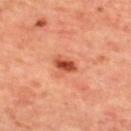• notes — total-body-photography surveillance lesion; no biopsy
• subject — female, in their mid-40s
• image — 15 mm crop, total-body photography
• site — the upper back
• tile lighting — cross-polarized
• size — about 2.5 mm